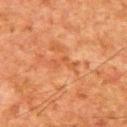The lesion was tiled from a total-body skin photograph and was not biopsied.
Longest diameter approximately 3.5 mm.
A male patient in their mid- to late 60s.
The lesion-visualizer software estimated a mean CIELAB color near L≈45 a*≈25 b*≈36 and a lesion–skin lightness drop of about 6. The analysis additionally found a nevus-likeness score of about 0/100 and lesion-presence confidence of about 85/100.
Imaged with cross-polarized lighting.
A 15 mm crop from a total-body photograph taken for skin-cancer surveillance.
The lesion is on the upper back.